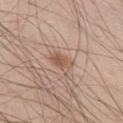image source: ~15 mm crop, total-body skin-cancer survey
lesion diameter: ~3 mm (longest diameter)
patient: male, roughly 50 years of age
site: the left thigh
lighting: white-light illumination